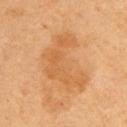  biopsy_status: not biopsied; imaged during a skin examination
  lesion_size:
    long_diameter_mm_approx: 7.0
  lighting: cross-polarized
  automated_metrics:
    cielab_L: 60
    cielab_a: 23
    cielab_b: 43
    vs_skin_darker_L: 7.0
    vs_skin_contrast_norm: 5.5
  site: back
  image:
    source: total-body photography crop
    field_of_view_mm: 15
  patient:
    sex: female
    age_approx: 65